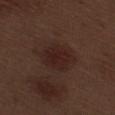The recorded lesion diameter is about 4.5 mm. A region of skin cropped from a whole-body photographic capture, roughly 15 mm wide. A male subject, about 70 years old. This is a white-light tile. Automated tile analysis of the lesion measured a footprint of about 13 mm² and a symmetry-axis asymmetry near 0.15. The analysis additionally found a mean CIELAB color near L≈23 a*≈17 b*≈20 and a normalized border contrast of about 7. The analysis additionally found a border-irregularity rating of about 1.5/10, internal color variation of about 3 on a 0–10 scale, and a peripheral color-asymmetry measure near 1. From the right thigh.